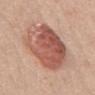<tbp_lesion>
<biopsy_status>not biopsied; imaged during a skin examination</biopsy_status>
<image>
  <source>total-body photography crop</source>
  <field_of_view_mm>15</field_of_view_mm>
</image>
<site>abdomen</site>
<automated_metrics>
  <area_mm2_approx>33.0</area_mm2_approx>
  <shape_asymmetry>0.1</shape_asymmetry>
  <cielab_L>55</cielab_L>
  <cielab_a>25</cielab_a>
  <cielab_b>28</cielab_b>
  <vs_skin_darker_L>13.0</vs_skin_darker_L>
  <vs_skin_contrast_norm>8.5</vs_skin_contrast_norm>
</automated_metrics>
<lesion_size>
  <long_diameter_mm_approx>6.5</long_diameter_mm_approx>
</lesion_size>
<patient>
  <sex>female</sex>
  <age_approx>45</age_approx>
</patient>
<lighting>white-light</lighting>
</tbp_lesion>